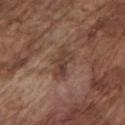biopsy status=total-body-photography surveillance lesion; no biopsy | diameter=≈4 mm | imaging modality=~15 mm crop, total-body skin-cancer survey | body site=the left upper arm | patient=male, aged 73–77 | lighting=white-light.